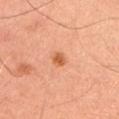Clinical impression:
Captured during whole-body skin photography for melanoma surveillance; the lesion was not biopsied.
Image and clinical context:
Located on the right upper arm. A 15 mm close-up tile from a total-body photography series done for melanoma screening. A male subject approximately 45 years of age.